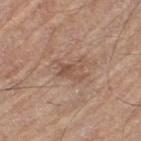Q: Is there a histopathology result?
A: total-body-photography surveillance lesion; no biopsy
Q: Patient demographics?
A: male, approximately 70 years of age
Q: Lesion location?
A: the left thigh
Q: How was the tile lit?
A: white-light illumination
Q: How was this image acquired?
A: ~15 mm tile from a whole-body skin photo
Q: What did automated image analysis measure?
A: lesion-presence confidence of about 100/100
Q: What is the lesion's diameter?
A: ~3 mm (longest diameter)